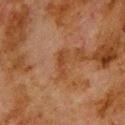notes: catalogued during a skin exam; not biopsied
imaging modality: total-body-photography crop, ~15 mm field of view
site: the upper back
automated lesion analysis: a lesion-detection confidence of about 100/100
patient: male, aged 78 to 82
illumination: cross-polarized illumination